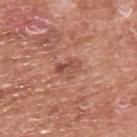The lesion was tiled from a total-body skin photograph and was not biopsied. The patient is a male approximately 75 years of age. The lesion is located on the upper back. An algorithmic analysis of the crop reported a mean CIELAB color near L≈51 a*≈25 b*≈29 and a normalized border contrast of about 6. It also reported border irregularity of about 4 on a 0–10 scale and a peripheral color-asymmetry measure near 1.5. It also reported a nevus-likeness score of about 0/100 and lesion-presence confidence of about 100/100. Measured at roughly 3.5 mm in maximum diameter. Captured under white-light illumination. A 15 mm close-up tile from a total-body photography series done for melanoma screening.